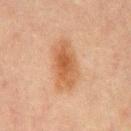Captured during whole-body skin photography for melanoma surveillance; the lesion was not biopsied.
A male subject, aged 63–67.
A lesion tile, about 15 mm wide, cut from a 3D total-body photograph.
Imaged with cross-polarized lighting.
On the mid back.
Longest diameter approximately 6 mm.